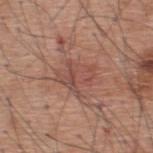Findings:
– image source — ~15 mm tile from a whole-body skin photo
– subject — male, aged around 60
– TBP lesion metrics — border irregularity of about 5.5 on a 0–10 scale, a color-variation rating of about 4.5/10, and radial color variation of about 1.5; an automated nevus-likeness rating near 0 out of 100 and lesion-presence confidence of about 75/100
– location — the upper back
– lighting — white-light illumination
– diameter — about 3.5 mm